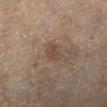Case summary:
* follow-up · no biopsy performed (imaged during a skin exam)
* imaging modality · ~15 mm crop, total-body skin-cancer survey
* location · the left lower leg
* patient · female, aged around 60
* size · about 2.5 mm
* lighting · cross-polarized illumination